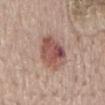{
  "biopsy_status": "not biopsied; imaged during a skin examination",
  "patient": {
    "sex": "female",
    "age_approx": 65
  },
  "lighting": "white-light",
  "lesion_size": {
    "long_diameter_mm_approx": 5.0
  },
  "automated_metrics": {
    "area_mm2_approx": 14.0,
    "eccentricity": 0.65,
    "shape_asymmetry": 0.1,
    "nevus_likeness_0_100": 95,
    "lesion_detection_confidence_0_100": 100
  },
  "site": "back",
  "image": {
    "source": "total-body photography crop",
    "field_of_view_mm": 15
  }
}